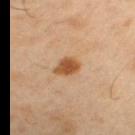| feature | finding |
|---|---|
| workup | imaged on a skin check; not biopsied |
| lesion size | ~3.5 mm (longest diameter) |
| illumination | cross-polarized |
| imaging modality | ~15 mm crop, total-body skin-cancer survey |
| location | the arm |
| patient | male, aged around 45 |
| image-analysis metrics | an average lesion color of about L≈50 a*≈21 b*≈36 (CIELAB) and a normalized lesion–skin contrast near 9.5; a border-irregularity rating of about 2.5/10 and a peripheral color-asymmetry measure near 1 |